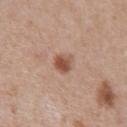follow-up=total-body-photography surveillance lesion; no biopsy | size=~2.5 mm (longest diameter) | patient=male, in their mid- to late 50s | location=the chest | image source=~15 mm crop, total-body skin-cancer survey | illumination=white-light | automated lesion analysis=a lesion area of about 4.5 mm², an eccentricity of roughly 0.7, and a symmetry-axis asymmetry near 0.2; a nevus-likeness score of about 90/100 and lesion-presence confidence of about 100/100.